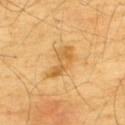Case summary:
* body site · the upper back
* illumination · cross-polarized
* image source · 15 mm crop, total-body photography
* TBP lesion metrics · an area of roughly 6.5 mm², an eccentricity of roughly 0.9, and two-axis asymmetry of about 0.4; an average lesion color of about L≈63 a*≈21 b*≈49 (CIELAB), roughly 10 lightness units darker than nearby skin, and a normalized lesion–skin contrast near 6.5; border irregularity of about 5 on a 0–10 scale, internal color variation of about 3 on a 0–10 scale, and peripheral color asymmetry of about 1; a nevus-likeness score of about 0/100
* diameter · about 4.5 mm
* patient · male, approximately 65 years of age